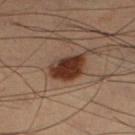<record>
  <biopsy_status>not biopsied; imaged during a skin examination</biopsy_status>
  <lesion_size>
    <long_diameter_mm_approx>4.0</long_diameter_mm_approx>
  </lesion_size>
  <patient>
    <sex>male</sex>
    <age_approx>55</age_approx>
  </patient>
  <automated_metrics>
    <area_mm2_approx>11.0</area_mm2_approx>
    <eccentricity>0.55</eccentricity>
    <shape_asymmetry>0.2</shape_asymmetry>
    <border_irregularity_0_10>2.0</border_irregularity_0_10>
  </automated_metrics>
  <site>right lower leg</site>
  <lighting>cross-polarized</lighting>
  <image>
    <source>total-body photography crop</source>
    <field_of_view_mm>15</field_of_view_mm>
  </image>
</record>